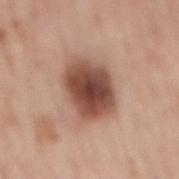Findings:
• biopsy status: catalogued during a skin exam; not biopsied
• acquisition: ~15 mm tile from a whole-body skin photo
• site: the back
• illumination: white-light illumination
• size: ~5.5 mm (longest diameter)
• subject: male, approximately 70 years of age